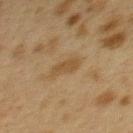biopsy status: imaged on a skin check; not biopsied
illumination: cross-polarized illumination
subject: female, aged 38 to 42
TBP lesion metrics: a footprint of about 4.5 mm², a shape eccentricity near 0.9, and a shape-asymmetry score of about 0.2 (0 = symmetric); border irregularity of about 3 on a 0–10 scale; an automated nevus-likeness rating near 0 out of 100 and a detector confidence of about 100 out of 100 that the crop contains a lesion
lesion size: about 3.5 mm
image: ~15 mm crop, total-body skin-cancer survey
anatomic site: the upper back